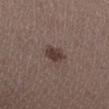biopsy status: total-body-photography surveillance lesion; no biopsy | image-analysis metrics: a border-irregularity rating of about 2/10, internal color variation of about 2 on a 0–10 scale, and peripheral color asymmetry of about 0.5 | subject: female, aged around 30 | site: the leg | lighting: white-light illumination | image: total-body-photography crop, ~15 mm field of view.